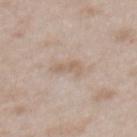Case summary:
– biopsy status: no biopsy performed (imaged during a skin exam)
– subject: female, in their 30s
– body site: the mid back
– lighting: white-light
– imaging modality: total-body-photography crop, ~15 mm field of view
– diameter: ≈3.5 mm